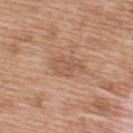The lesion was photographed on a routine skin check and not biopsied; there is no pathology result.